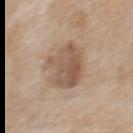follow-up: no biopsy performed (imaged during a skin exam) | body site: the upper back | lesion size: ≈5.5 mm | lighting: white-light illumination | acquisition: 15 mm crop, total-body photography | subject: male, aged 83 or older.